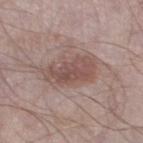Case summary:
- workup · total-body-photography surveillance lesion; no biopsy